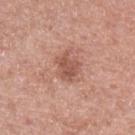This lesion was catalogued during total-body skin photography and was not selected for biopsy.
The lesion is located on the left forearm.
A lesion tile, about 15 mm wide, cut from a 3D total-body photograph.
A male subject, aged 58–62.
Measured at roughly 3.5 mm in maximum diameter.
Imaged with white-light lighting.